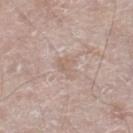acquisition: 15 mm crop, total-body photography
anatomic site: the right lower leg
illumination: white-light illumination
image-analysis metrics: a mean CIELAB color near L≈61 a*≈15 b*≈24 and roughly 6 lightness units darker than nearby skin; an automated nevus-likeness rating near 0 out of 100 and a detector confidence of about 90 out of 100 that the crop contains a lesion
patient: male, aged approximately 65
size: ≈3 mm